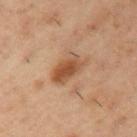follow-up = total-body-photography surveillance lesion; no biopsy | subject = male, aged 53–57 | imaging modality = ~15 mm tile from a whole-body skin photo | body site = the left upper arm.